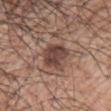| field | value |
|---|---|
| notes | total-body-photography surveillance lesion; no biopsy |
| acquisition | ~15 mm crop, total-body skin-cancer survey |
| size | about 3.5 mm |
| automated metrics | an outline eccentricity of about 0.65 (0 = round, 1 = elongated); an average lesion color of about L≈43 a*≈18 b*≈23 (CIELAB) and a normalized lesion–skin contrast near 9.5; a border-irregularity rating of about 3.5/10, a color-variation rating of about 3.5/10, and a peripheral color-asymmetry measure near 1.5 |
| patient | male, roughly 70 years of age |
| body site | the arm |